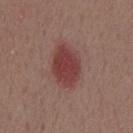This lesion was catalogued during total-body skin photography and was not selected for biopsy. A male patient, roughly 55 years of age. Longest diameter approximately 5.5 mm. An algorithmic analysis of the crop reported a lesion area of about 14 mm², an outline eccentricity of about 0.75 (0 = round, 1 = elongated), and a symmetry-axis asymmetry near 0.15. And it measured a lesion color around L≈41 a*≈25 b*≈22 in CIELAB, about 11 CIELAB-L* units darker than the surrounding skin, and a lesion-to-skin contrast of about 8.5 (normalized; higher = more distinct). It also reported a nevus-likeness score of about 100/100. The lesion is located on the chest. A close-up tile cropped from a whole-body skin photograph, about 15 mm across. Captured under white-light illumination.